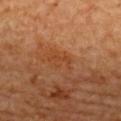No biopsy was performed on this lesion — it was imaged during a full skin examination and was not determined to be concerning. Cropped from a total-body skin-imaging series; the visible field is about 15 mm. The recorded lesion diameter is about 3 mm. A female subject. The lesion is on the upper back.